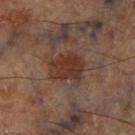<case>
  <biopsy_status>not biopsied; imaged during a skin examination</biopsy_status>
  <site>right lower leg</site>
  <lesion_size>
    <long_diameter_mm_approx>4.0</long_diameter_mm_approx>
  </lesion_size>
  <image>
    <source>total-body photography crop</source>
    <field_of_view_mm>15</field_of_view_mm>
  </image>
</case>